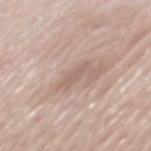The lesion was tiled from a total-body skin photograph and was not biopsied.
The patient is a male aged 78 to 82.
This is a white-light tile.
A close-up tile cropped from a whole-body skin photograph, about 15 mm across.
Located on the back.